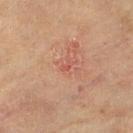Imaged during a routine full-body skin examination; the lesion was not biopsied and no histopathology is available. A 15 mm close-up tile from a total-body photography series done for melanoma screening. Captured under cross-polarized illumination. A female patient, aged 78–82. Located on the right lower leg.